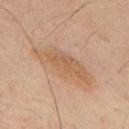Recorded during total-body skin imaging; not selected for excision or biopsy. A male patient aged approximately 50. The total-body-photography lesion software estimated a lesion area of about 15 mm², an outline eccentricity of about 0.95 (0 = round, 1 = elongated), and two-axis asymmetry of about 0.3. The analysis additionally found a lesion color around L≈52 a*≈17 b*≈31 in CIELAB, a lesion–skin lightness drop of about 7, and a lesion-to-skin contrast of about 6 (normalized; higher = more distinct). From the upper back. Imaged with cross-polarized lighting. The recorded lesion diameter is about 7.5 mm. A 15 mm close-up extracted from a 3D total-body photography capture.